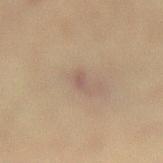  biopsy_status: not biopsied; imaged during a skin examination
  image:
    source: total-body photography crop
    field_of_view_mm: 15
  site: lower back
  patient:
    sex: female
    age_approx: 80